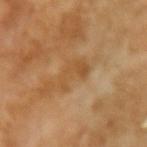biopsy status = catalogued during a skin exam; not biopsied | patient = female, approximately 70 years of age | automated lesion analysis = a classifier nevus-likeness of about 0/100 | diameter = about 4 mm | body site = the right forearm | lighting = cross-polarized | image = ~15 mm tile from a whole-body skin photo.